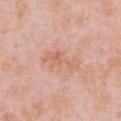- biopsy status: catalogued during a skin exam; not biopsied
- acquisition: ~15 mm crop, total-body skin-cancer survey
- diameter: about 4.5 mm
- anatomic site: the chest
- patient: female, aged approximately 40
- lighting: white-light